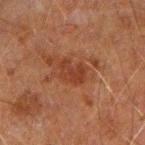image:
  source: total-body photography crop
  field_of_view_mm: 15
site: left arm
patient:
  sex: male
  age_approx: 60